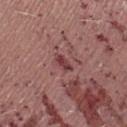Imaged during a routine full-body skin examination; the lesion was not biopsied and no histopathology is available.
A female patient in their 20s.
Located on the right lower leg.
The lesion's longest dimension is about 2.5 mm.
Captured under white-light illumination.
A close-up tile cropped from a whole-body skin photograph, about 15 mm across.